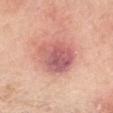Q: What lighting was used for the tile?
A: white-light
Q: How large is the lesion?
A: ~5.5 mm (longest diameter)
Q: Where on the body is the lesion?
A: the right forearm
Q: How was this image acquired?
A: ~15 mm tile from a whole-body skin photo
Q: Automated lesion metrics?
A: a lesion area of about 20 mm²; an average lesion color of about L≈60 a*≈28 b*≈25 (CIELAB), about 13 CIELAB-L* units darker than the surrounding skin, and a normalized border contrast of about 8.5; a border-irregularity rating of about 1.5/10, a within-lesion color-variation index near 7/10, and peripheral color asymmetry of about 2; a classifier nevus-likeness of about 0/100
Q: Patient demographics?
A: female, approximately 70 years of age